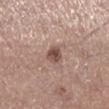image source: ~15 mm crop, total-body skin-cancer survey
subject: male, aged 53 to 57
size: ~2.5 mm (longest diameter)
illumination: white-light
site: the left lower leg
image-analysis metrics: an average lesion color of about L≈49 a*≈19 b*≈23 (CIELAB), about 13 CIELAB-L* units darker than the surrounding skin, and a normalized lesion–skin contrast near 9; lesion-presence confidence of about 100/100